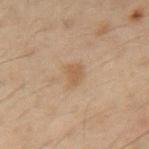<case>
<biopsy_status>not biopsied; imaged during a skin examination</biopsy_status>
<lighting>cross-polarized</lighting>
<lesion_size>
  <long_diameter_mm_approx>2.5</long_diameter_mm_approx>
</lesion_size>
<patient>
  <sex>male</sex>
  <age_approx>50</age_approx>
</patient>
<site>mid back</site>
<image>
  <source>total-body photography crop</source>
  <field_of_view_mm>15</field_of_view_mm>
</image>
</case>